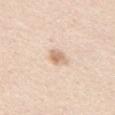{
  "biopsy_status": "not biopsied; imaged during a skin examination",
  "automated_metrics": {
    "cielab_L": 70,
    "cielab_a": 17,
    "cielab_b": 31,
    "vs_skin_darker_L": 12.0,
    "vs_skin_contrast_norm": 7.0
  },
  "lesion_size": {
    "long_diameter_mm_approx": 2.5
  },
  "lighting": "white-light",
  "image": {
    "source": "total-body photography crop",
    "field_of_view_mm": 15
  },
  "patient": {
    "sex": "male",
    "age_approx": 45
  },
  "site": "chest"
}